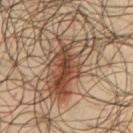Recorded during total-body skin imaging; not selected for excision or biopsy. This image is a 15 mm lesion crop taken from a total-body photograph. The lesion-visualizer software estimated a lesion area of about 23 mm², a shape eccentricity near 0.85, and a symmetry-axis asymmetry near 0.4. The software also gave a border-irregularity rating of about 6.5/10 and peripheral color asymmetry of about 3.5. It also reported an automated nevus-likeness rating near 100 out of 100 and a detector confidence of about 70 out of 100 that the crop contains a lesion. About 8.5 mm across. The lesion is on the chest. The patient is a male aged 63–67.